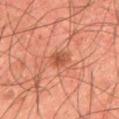This lesion was catalogued during total-body skin photography and was not selected for biopsy.
Imaged with cross-polarized lighting.
The lesion is on the upper back.
About 2.5 mm across.
Automated tile analysis of the lesion measured a lesion color around L≈50 a*≈29 b*≈35 in CIELAB, about 10 CIELAB-L* units darker than the surrounding skin, and a normalized lesion–skin contrast near 7.
A region of skin cropped from a whole-body photographic capture, roughly 15 mm wide.
A male subject, in their mid- to late 40s.